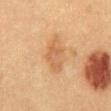Case summary:
* follow-up · no biopsy performed (imaged during a skin exam)
* acquisition · ~15 mm crop, total-body skin-cancer survey
* subject · male, approximately 50 years of age
* diameter · ≈5 mm
* automated metrics · a classifier nevus-likeness of about 25/100
* site · the abdomen